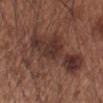Assessment:
The lesion was tiled from a total-body skin photograph and was not biopsied.
Clinical summary:
A 15 mm close-up extracted from a 3D total-body photography capture. This is a white-light tile. A male patient aged 53 to 57. The lesion is located on the arm. Approximately 10.5 mm at its widest.